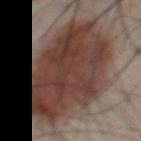<case>
<biopsy_status>not biopsied; imaged during a skin examination</biopsy_status>
<lesion_size>
  <long_diameter_mm_approx>12.0</long_diameter_mm_approx>
</lesion_size>
<site>front of the torso</site>
<patient>
  <sex>male</sex>
  <age_approx>55</age_approx>
</patient>
<image>
  <source>total-body photography crop</source>
  <field_of_view_mm>15</field_of_view_mm>
</image>
<automated_metrics>
  <shape_asymmetry>0.15</shape_asymmetry>
  <cielab_L>39</cielab_L>
  <cielab_a>18</cielab_a>
  <cielab_b>23</cielab_b>
  <vs_skin_contrast_norm>11.0</vs_skin_contrast_norm>
  <border_irregularity_0_10>2.5</border_irregularity_0_10>
  <color_variation_0_10>5.5</color_variation_0_10>
  <peripheral_color_asymmetry>2.0</peripheral_color_asymmetry>
  <nevus_likeness_0_100>100</nevus_likeness_0_100>
  <lesion_detection_confidence_0_100>50</lesion_detection_confidence_0_100>
</automated_metrics>
</case>